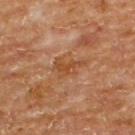Captured during whole-body skin photography for melanoma surveillance; the lesion was not biopsied.
The subject is a male aged around 65.
From the upper back.
A region of skin cropped from a whole-body photographic capture, roughly 15 mm wide.
Longest diameter approximately 4 mm.
Captured under cross-polarized illumination.
The lesion-visualizer software estimated an area of roughly 5.5 mm² and an eccentricity of roughly 0.85. The analysis additionally found a lesion color around L≈43 a*≈22 b*≈34 in CIELAB, a lesion–skin lightness drop of about 7, and a lesion-to-skin contrast of about 6.5 (normalized; higher = more distinct). The analysis additionally found a classifier nevus-likeness of about 0/100 and lesion-presence confidence of about 100/100.